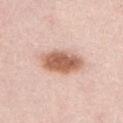The lesion was photographed on a routine skin check and not biopsied; there is no pathology result. Approximately 6 mm at its widest. Cropped from a total-body skin-imaging series; the visible field is about 15 mm. The subject is a female about 60 years old. The lesion is located on the abdomen.